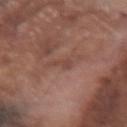The lesion was tiled from a total-body skin photograph and was not biopsied. The tile uses white-light illumination. The patient is a male about 75 years old. The lesion is on the arm. A 15 mm close-up extracted from a 3D total-body photography capture. Approximately 3 mm at its widest.